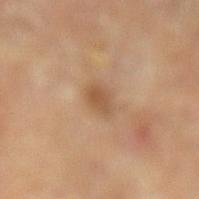biopsy status: catalogued during a skin exam; not biopsied
patient: roughly 55 years of age
image source: 15 mm crop, total-body photography
body site: the left lower leg
lesion size: ≈2.5 mm